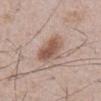biopsy_status: not biopsied; imaged during a skin examination
image:
  source: total-body photography crop
  field_of_view_mm: 15
lighting: white-light
lesion_size:
  long_diameter_mm_approx: 4.0
patient:
  sex: male
  age_approx: 55